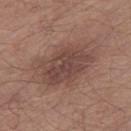Part of a total-body skin-imaging series; this lesion was reviewed on a skin check and was not flagged for biopsy.
An algorithmic analysis of the crop reported a border-irregularity rating of about 3/10 and a color-variation rating of about 3.5/10. It also reported a nevus-likeness score of about 15/100 and a detector confidence of about 100 out of 100 that the crop contains a lesion.
The lesion is located on the right thigh.
This is a white-light tile.
A male subject, aged around 65.
A 15 mm close-up extracted from a 3D total-body photography capture.